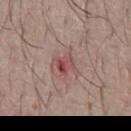Clinical impression:
This lesion was catalogued during total-body skin photography and was not selected for biopsy.
Context:
The tile uses white-light illumination. The subject is a male roughly 65 years of age. Cropped from a whole-body photographic skin survey; the tile spans about 15 mm. The lesion is on the abdomen. Approximately 3 mm at its widest. The lesion-visualizer software estimated a lesion area of about 5 mm², an outline eccentricity of about 0.65 (0 = round, 1 = elongated), and two-axis asymmetry of about 0.45. It also reported a mean CIELAB color near L≈49 a*≈24 b*≈21, roughly 9 lightness units darker than nearby skin, and a lesion-to-skin contrast of about 7 (normalized; higher = more distinct).